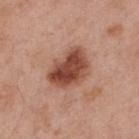follow-up: total-body-photography surveillance lesion; no biopsy
acquisition: total-body-photography crop, ~15 mm field of view
patient: male, aged around 55
body site: the upper back